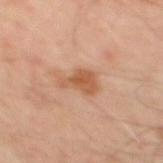Assessment: No biopsy was performed on this lesion — it was imaged during a full skin examination and was not determined to be concerning. Acquisition and patient details: The total-body-photography lesion software estimated an average lesion color of about L≈56 a*≈23 b*≈34 (CIELAB), a lesion–skin lightness drop of about 10, and a lesion-to-skin contrast of about 7.5 (normalized; higher = more distinct). A region of skin cropped from a whole-body photographic capture, roughly 15 mm wide. A male subject, in their 50s. This is a cross-polarized tile. On the upper back. About 4 mm across.